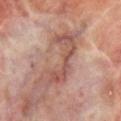site: the head or neck | tile lighting: cross-polarized | patient: female, approximately 70 years of age | acquisition: total-body-photography crop, ~15 mm field of view | diameter: ~8 mm (longest diameter) | image-analysis metrics: an average lesion color of about L≈45 a*≈20 b*≈22 (CIELAB), a lesion–skin lightness drop of about 9, and a lesion-to-skin contrast of about 7 (normalized; higher = more distinct).